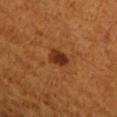• biopsy status: imaged on a skin check; not biopsied
• lesion diameter: about 3 mm
• tile lighting: cross-polarized
• location: the arm
• imaging modality: total-body-photography crop, ~15 mm field of view
• subject: female, roughly 55 years of age